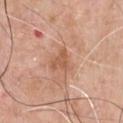The lesion was photographed on a routine skin check and not biopsied; there is no pathology result. The lesion is on the chest. A 15 mm close-up tile from a total-body photography series done for melanoma screening. A male subject, roughly 60 years of age.